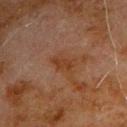{
  "biopsy_status": "not biopsied; imaged during a skin examination",
  "site": "left upper arm",
  "lesion_size": {
    "long_diameter_mm_approx": 3.0
  },
  "patient": {
    "sex": "male",
    "age_approx": 80
  },
  "image": {
    "source": "total-body photography crop",
    "field_of_view_mm": 15
  },
  "automated_metrics": {
    "area_mm2_approx": 4.0,
    "eccentricity": 0.85,
    "shape_asymmetry": 0.25,
    "cielab_L": 29,
    "cielab_a": 19,
    "cielab_b": 27,
    "vs_skin_darker_L": 5.0,
    "vs_skin_contrast_norm": 6.5
  }
}